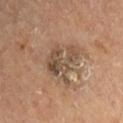  biopsy_status: not biopsied; imaged during a skin examination
  site: right upper arm
  patient:
    sex: male
    age_approx: 65
  lighting: cross-polarized
  image:
    source: total-body photography crop
    field_of_view_mm: 15
  lesion_size:
    long_diameter_mm_approx: 5.0